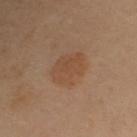Clinical impression: The lesion was photographed on a routine skin check and not biopsied; there is no pathology result. Context: Automated image analysis of the tile measured an area of roughly 11 mm², a shape eccentricity near 0.65, and a shape-asymmetry score of about 0.2 (0 = symmetric). The software also gave an average lesion color of about L≈40 a*≈16 b*≈27 (CIELAB), about 5 CIELAB-L* units darker than the surrounding skin, and a normalized border contrast of about 5.5. The software also gave a classifier nevus-likeness of about 50/100. About 4 mm across. A region of skin cropped from a whole-body photographic capture, roughly 15 mm wide. The tile uses cross-polarized illumination. A female subject, approximately 60 years of age. The lesion is located on the upper back.